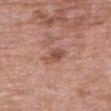Assessment: No biopsy was performed on this lesion — it was imaged during a full skin examination and was not determined to be concerning. Context: A female subject aged around 70. From the arm. About 3 mm across. This image is a 15 mm lesion crop taken from a total-body photograph.